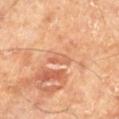Imaged during a routine full-body skin examination; the lesion was not biopsied and no histopathology is available.
Imaged with cross-polarized lighting.
A close-up tile cropped from a whole-body skin photograph, about 15 mm across.
A male patient, roughly 70 years of age.
Located on the left lower leg.
The lesion's longest dimension is about 3 mm.